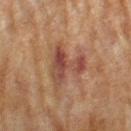{
  "biopsy_status": "not biopsied; imaged during a skin examination",
  "automated_metrics": {
    "eccentricity": 0.35,
    "shape_asymmetry": 0.75,
    "cielab_L": 39,
    "cielab_a": 21,
    "cielab_b": 24,
    "vs_skin_darker_L": 9.0,
    "vs_skin_contrast_norm": 8.0,
    "nevus_likeness_0_100": 0,
    "lesion_detection_confidence_0_100": 100
  },
  "patient": {
    "sex": "female",
    "age_approx": 80
  },
  "lighting": "cross-polarized",
  "site": "left forearm",
  "lesion_size": {
    "long_diameter_mm_approx": 5.0
  },
  "image": {
    "source": "total-body photography crop",
    "field_of_view_mm": 15
  }
}